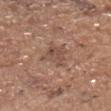Q: Was this lesion biopsied?
A: total-body-photography surveillance lesion; no biopsy
Q: Who is the patient?
A: male, in their 70s
Q: Where on the body is the lesion?
A: the chest
Q: How large is the lesion?
A: ~3.5 mm (longest diameter)
Q: What kind of image is this?
A: 15 mm crop, total-body photography